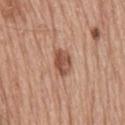The lesion was photographed on a routine skin check and not biopsied; there is no pathology result.
Located on the upper back.
A male subject aged approximately 80.
A 15 mm close-up tile from a total-body photography series done for melanoma screening.
About 3.5 mm across.